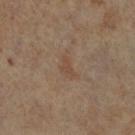Captured during whole-body skin photography for melanoma surveillance; the lesion was not biopsied.
The lesion is located on the left leg.
This image is a 15 mm lesion crop taken from a total-body photograph.
The subject is a female aged around 65.